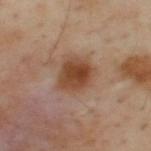workup: no biopsy performed (imaged during a skin exam) | subject: male, in their mid-50s | location: the back | image: total-body-photography crop, ~15 mm field of view.